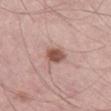diameter: ~3 mm (longest diameter) | image source: total-body-photography crop, ~15 mm field of view | site: the leg | lighting: white-light illumination | subject: male, roughly 55 years of age.